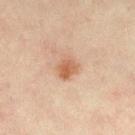An algorithmic analysis of the crop reported an area of roughly 5.5 mm² and an outline eccentricity of about 0.55 (0 = round, 1 = elongated). It also reported a mean CIELAB color near L≈53 a*≈19 b*≈30 and about 9 CIELAB-L* units darker than the surrounding skin. The analysis additionally found border irregularity of about 1.5 on a 0–10 scale, a within-lesion color-variation index near 4.5/10, and radial color variation of about 1.5. A female subject, in their mid-40s. Located on the leg. A region of skin cropped from a whole-body photographic capture, roughly 15 mm wide. Longest diameter approximately 2.5 mm. Captured under cross-polarized illumination.